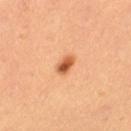Q: Was this lesion biopsied?
A: no biopsy performed (imaged during a skin exam)
Q: What is the imaging modality?
A: 15 mm crop, total-body photography
Q: Who is the patient?
A: female, aged around 35
Q: What lighting was used for the tile?
A: cross-polarized illumination
Q: What did automated image analysis measure?
A: a color-variation rating of about 6/10 and radial color variation of about 2; a nevus-likeness score of about 100/100 and lesion-presence confidence of about 100/100
Q: Where on the body is the lesion?
A: the leg